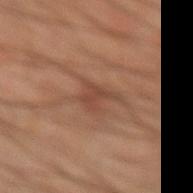Impression:
The lesion was photographed on a routine skin check and not biopsied; there is no pathology result.
Background:
On the right forearm. A male subject, in their mid- to late 40s. A 15 mm crop from a total-body photograph taken for skin-cancer surveillance. Measured at roughly 3.5 mm in maximum diameter.